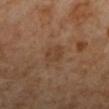workup=imaged on a skin check; not biopsied
imaging modality=~15 mm tile from a whole-body skin photo
diameter=~2.5 mm (longest diameter)
tile lighting=cross-polarized
anatomic site=the left lower leg
subject=female, aged approximately 50